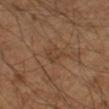workup: catalogued during a skin exam; not biopsied | illumination: cross-polarized | subject: male, in their mid- to late 60s | image source: ~15 mm tile from a whole-body skin photo | automated metrics: a footprint of about 4.5 mm² and a shape eccentricity near 0.55; roughly 5 lightness units darker than nearby skin and a normalized lesion–skin contrast near 4.5; a border-irregularity rating of about 2.5/10, internal color variation of about 3 on a 0–10 scale, and a peripheral color-asymmetry measure near 1.5; an automated nevus-likeness rating near 0 out of 100 and lesion-presence confidence of about 95/100 | site: the left arm.